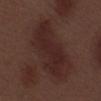| key | value |
|---|---|
| follow-up | imaged on a skin check; not biopsied |
| anatomic site | the left lower leg |
| lighting | white-light illumination |
| size | ~9 mm (longest diameter) |
| subject | male, aged approximately 70 |
| image | total-body-photography crop, ~15 mm field of view |